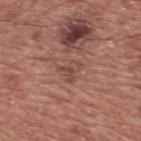Impression:
Captured during whole-body skin photography for melanoma surveillance; the lesion was not biopsied.
Clinical summary:
Longest diameter approximately 2.5 mm. Captured under white-light illumination. On the back. A 15 mm close-up extracted from a 3D total-body photography capture. A male subject, in their mid-70s.